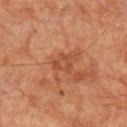The lesion was tiled from a total-body skin photograph and was not biopsied.
Located on the chest.
Automated image analysis of the tile measured a lesion area of about 4.5 mm² and a symmetry-axis asymmetry near 0.35. The analysis additionally found a border-irregularity rating of about 4/10, a color-variation rating of about 3/10, and radial color variation of about 1.
A close-up tile cropped from a whole-body skin photograph, about 15 mm across.
Measured at roughly 2.5 mm in maximum diameter.
A male patient in their mid-60s.